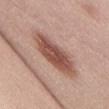Impression:
The lesion was photographed on a routine skin check and not biopsied; there is no pathology result.
Image and clinical context:
Longest diameter approximately 8 mm. Imaged with white-light lighting. Automated image analysis of the tile measured a footprint of about 17 mm², an outline eccentricity of about 0.95 (0 = round, 1 = elongated), and a shape-asymmetry score of about 0.2 (0 = symmetric). And it measured a border-irregularity rating of about 3.5/10, a within-lesion color-variation index near 4/10, and radial color variation of about 1.5. The lesion is on the abdomen. A female patient, about 40 years old. A lesion tile, about 15 mm wide, cut from a 3D total-body photograph.